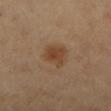workup: no biopsy performed (imaged during a skin exam); image: total-body-photography crop, ~15 mm field of view; location: the left lower leg; patient: female, aged 58 to 62; lighting: cross-polarized illumination.